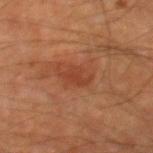This lesion was catalogued during total-body skin photography and was not selected for biopsy. The lesion is located on the right thigh. Imaged with cross-polarized lighting. The lesion's longest dimension is about 3.5 mm. A male subject in their mid- to late 60s. This image is a 15 mm lesion crop taken from a total-body photograph.